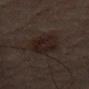image source: ~15 mm crop, total-body skin-cancer survey | subject: male, in their mid- to late 80s or older | body site: the left thigh.